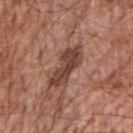<lesion>
  <lesion_size>
    <long_diameter_mm_approx>6.0</long_diameter_mm_approx>
  </lesion_size>
  <lighting>white-light</lighting>
  <image>
    <source>total-body photography crop</source>
    <field_of_view_mm>15</field_of_view_mm>
  </image>
  <patient>
    <sex>male</sex>
    <age_approx>55</age_approx>
  </patient>
  <site>right upper arm</site>
</lesion>